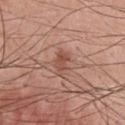Impression: No biopsy was performed on this lesion — it was imaged during a full skin examination and was not determined to be concerning. Image and clinical context: This is a white-light tile. The lesion is located on the chest. A lesion tile, about 15 mm wide, cut from a 3D total-body photograph. A male patient in their mid- to late 50s. Measured at roughly 3 mm in maximum diameter.